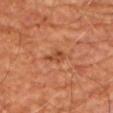Findings:
– follow-up — no biopsy performed (imaged during a skin exam)
– imaging modality — 15 mm crop, total-body photography
– subject — male, about 65 years old
– location — the left upper arm
– tile lighting — cross-polarized